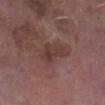Notes:
• biopsy status: total-body-photography surveillance lesion; no biopsy
• tile lighting: white-light
• site: the left lower leg
• subject: male, roughly 75 years of age
• image source: ~15 mm tile from a whole-body skin photo
• lesion diameter: ~4 mm (longest diameter)